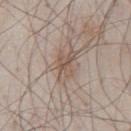workup: no biopsy performed (imaged during a skin exam)
lighting: white-light
image: total-body-photography crop, ~15 mm field of view
subject: male, aged approximately 80
image-analysis metrics: an area of roughly 7.5 mm², an eccentricity of roughly 0.75, and a shape-asymmetry score of about 0.25 (0 = symmetric); a border-irregularity rating of about 3.5/10 and a color-variation rating of about 4.5/10; a classifier nevus-likeness of about 0/100
site: the chest
lesion diameter: about 4 mm